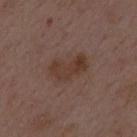Q: Was this lesion biopsied?
A: catalogued during a skin exam; not biopsied
Q: How large is the lesion?
A: about 4.5 mm
Q: What kind of image is this?
A: ~15 mm crop, total-body skin-cancer survey
Q: Illumination type?
A: white-light illumination
Q: What are the patient's age and sex?
A: male, approximately 50 years of age
Q: What is the anatomic site?
A: the mid back